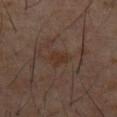workup = no biopsy performed (imaged during a skin exam); patient = male, aged around 60; automated metrics = a lesion-detection confidence of about 100/100; acquisition = total-body-photography crop, ~15 mm field of view; anatomic site = the abdomen; diameter = ~3 mm (longest diameter).